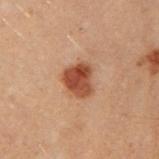<lesion>
  <biopsy_status>not biopsied; imaged during a skin examination</biopsy_status>
  <site>left arm</site>
  <patient>
    <sex>female</sex>
    <age_approx>30</age_approx>
  </patient>
  <lighting>cross-polarized</lighting>
  <image>
    <source>total-body photography crop</source>
    <field_of_view_mm>15</field_of_view_mm>
  </image>
  <lesion_size>
    <long_diameter_mm_approx>3.5</long_diameter_mm_approx>
  </lesion_size>
</lesion>